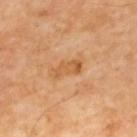Imaged during a routine full-body skin examination; the lesion was not biopsied and no histopathology is available.
Located on the upper back.
The patient is a male aged around 55.
This image is a 15 mm lesion crop taken from a total-body photograph.